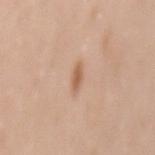follow-up — no biopsy performed (imaged during a skin exam)
diameter — about 2.5 mm
image source — total-body-photography crop, ~15 mm field of view
TBP lesion metrics — an area of roughly 2.5 mm² and a shape-asymmetry score of about 0.45 (0 = symmetric); about 10 CIELAB-L* units darker than the surrounding skin and a lesion-to-skin contrast of about 7 (normalized; higher = more distinct); a border-irregularity index near 4/10, a within-lesion color-variation index near 1/10, and peripheral color asymmetry of about 0.5
subject — female, aged 48 to 52
site — the mid back